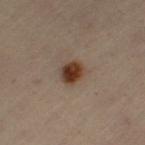<record>
  <lesion_size>
    <long_diameter_mm_approx>3.0</long_diameter_mm_approx>
  </lesion_size>
  <patient>
    <sex>female</sex>
    <age_approx>50</age_approx>
  </patient>
  <image>
    <source>total-body photography crop</source>
    <field_of_view_mm>15</field_of_view_mm>
  </image>
  <site>left thigh</site>
</record>